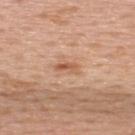Assessment: No biopsy was performed on this lesion — it was imaged during a full skin examination and was not determined to be concerning. Acquisition and patient details: A male patient, aged approximately 65. Measured at roughly 2.5 mm in maximum diameter. A 15 mm close-up tile from a total-body photography series done for melanoma screening. From the upper back. The lesion-visualizer software estimated a footprint of about 3.5 mm², an eccentricity of roughly 0.85, and a shape-asymmetry score of about 0.3 (0 = symmetric). And it measured a mean CIELAB color near L≈58 a*≈23 b*≈34. The analysis additionally found a border-irregularity rating of about 3/10, internal color variation of about 4.5 on a 0–10 scale, and radial color variation of about 1.5. It also reported a classifier nevus-likeness of about 0/100 and a lesion-detection confidence of about 100/100.